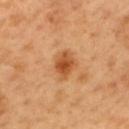Notes:
• biopsy status — catalogued during a skin exam; not biopsied
• patient — male, aged approximately 50
• image source — 15 mm crop, total-body photography
• site — the back